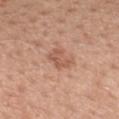Notes:
– notes — catalogued during a skin exam; not biopsied
– lesion size — ≈3.5 mm
– patient — male, aged 48 to 52
– body site — the mid back
– image — ~15 mm crop, total-body skin-cancer survey